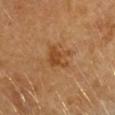| field | value |
|---|---|
| location | the head or neck |
| image source | ~15 mm tile from a whole-body skin photo |
| patient | female, aged approximately 65 |